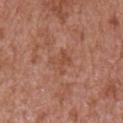Q: Was this lesion biopsied?
A: total-body-photography surveillance lesion; no biopsy
Q: What is the lesion's diameter?
A: about 2.5 mm
Q: Patient demographics?
A: male, in their mid-60s
Q: What kind of image is this?
A: ~15 mm tile from a whole-body skin photo
Q: Lesion location?
A: the right upper arm